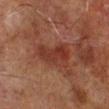Recorded during total-body skin imaging; not selected for excision or biopsy.
The lesion's longest dimension is about 5 mm.
Imaged with cross-polarized lighting.
A male patient, about 70 years old.
On the right lower leg.
A region of skin cropped from a whole-body photographic capture, roughly 15 mm wide.
Automated image analysis of the tile measured a border-irregularity rating of about 3.5/10 and a peripheral color-asymmetry measure near 1.5. The analysis additionally found a lesion-detection confidence of about 100/100.